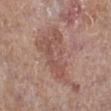Case summary:
- biopsy status · imaged on a skin check; not biopsied
- image · 15 mm crop, total-body photography
- body site · the leg
- patient · male, aged around 80